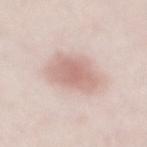subject: female, in their 40s | automated metrics: a footprint of about 14 mm², a shape eccentricity near 0.7, and two-axis asymmetry of about 0.2; a lesion-to-skin contrast of about 6.5 (normalized; higher = more distinct) | acquisition: total-body-photography crop, ~15 mm field of view | tile lighting: white-light | site: the mid back.